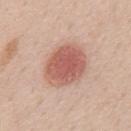Case summary:
– lighting — white-light
– acquisition — 15 mm crop, total-body photography
– diameter — about 5 mm
– patient — male, aged around 60
– anatomic site — the mid back
– TBP lesion metrics — an average lesion color of about L≈58 a*≈25 b*≈27 (CIELAB), roughly 13 lightness units darker than nearby skin, and a normalized lesion–skin contrast near 8; a border-irregularity rating of about 1/10; an automated nevus-likeness rating near 100 out of 100 and a detector confidence of about 100 out of 100 that the crop contains a lesion